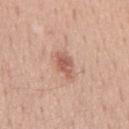biopsy status: catalogued during a skin exam; not biopsied | location: the back | subject: male, aged 48–52 | diameter: ≈3.5 mm | image-analysis metrics: a mean CIELAB color near L≈58 a*≈24 b*≈28, roughly 11 lightness units darker than nearby skin, and a lesion-to-skin contrast of about 7.5 (normalized; higher = more distinct) | illumination: white-light | image source: ~15 mm crop, total-body skin-cancer survey.